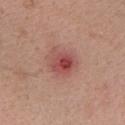Clinical impression: The lesion was tiled from a total-body skin photograph and was not biopsied. Image and clinical context: The lesion is on the head or neck. The subject is a female in their 30s. A region of skin cropped from a whole-body photographic capture, roughly 15 mm wide.